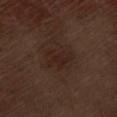notes: no biopsy performed (imaged during a skin exam) | patient: male, about 70 years old | automated metrics: a footprint of about 7.5 mm², an eccentricity of roughly 0.6, and a shape-asymmetry score of about 0.4 (0 = symmetric); an average lesion color of about L≈22 a*≈16 b*≈21 (CIELAB), roughly 5 lightness units darker than nearby skin, and a lesion-to-skin contrast of about 6 (normalized; higher = more distinct); a classifier nevus-likeness of about 5/100 and a detector confidence of about 100 out of 100 that the crop contains a lesion | site: the left thigh | image: ~15 mm tile from a whole-body skin photo | size: about 3.5 mm.